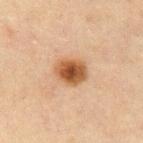The lesion was tiled from a total-body skin photograph and was not biopsied.
On the abdomen.
A close-up tile cropped from a whole-body skin photograph, about 15 mm across.
Captured under cross-polarized illumination.
Measured at roughly 3.5 mm in maximum diameter.
A male patient in their 70s.